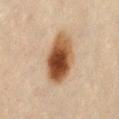The total-body-photography lesion software estimated a footprint of about 18 mm² and an eccentricity of roughly 0.8. The software also gave an average lesion color of about L≈45 a*≈18 b*≈31 (CIELAB). And it measured a nevus-likeness score of about 100/100 and a lesion-detection confidence of about 100/100. The subject is a female aged 43 to 47. About 6 mm across. Located on the abdomen. A region of skin cropped from a whole-body photographic capture, roughly 15 mm wide.